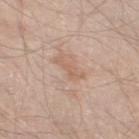{"biopsy_status": "not biopsied; imaged during a skin examination", "patient": {"sex": "male", "age_approx": 60}, "lighting": "white-light", "site": "left thigh", "image": {"source": "total-body photography crop", "field_of_view_mm": 15}, "automated_metrics": {"area_mm2_approx": 3.5, "eccentricity": 0.95, "shape_asymmetry": 0.5, "cielab_L": 61, "cielab_a": 18, "cielab_b": 30, "vs_skin_darker_L": 7.0, "nevus_likeness_0_100": 0, "lesion_detection_confidence_0_100": 100}}